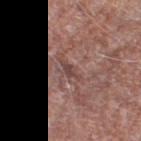Notes:
* notes · imaged on a skin check; not biopsied
* image-analysis metrics · a lesion–skin lightness drop of about 8 and a normalized border contrast of about 6.5
* tile lighting · white-light
* lesion diameter · ≈3 mm
* subject · male, approximately 80 years of age
* imaging modality · 15 mm crop, total-body photography
* body site · the right thigh